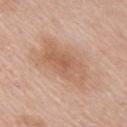Impression:
Imaged during a routine full-body skin examination; the lesion was not biopsied and no histopathology is available.
Acquisition and patient details:
The patient is a male roughly 65 years of age. Captured under white-light illumination. The recorded lesion diameter is about 6.5 mm. A close-up tile cropped from a whole-body skin photograph, about 15 mm across. Automated image analysis of the tile measured an eccentricity of roughly 0.85 and a shape-asymmetry score of about 0.25 (0 = symmetric). The analysis additionally found a mean CIELAB color near L≈60 a*≈21 b*≈32, about 8 CIELAB-L* units darker than the surrounding skin, and a normalized lesion–skin contrast near 6. And it measured an automated nevus-likeness rating near 5 out of 100 and a lesion-detection confidence of about 100/100. Located on the right upper arm.